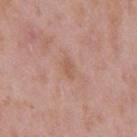The lesion was tiled from a total-body skin photograph and was not biopsied. A 15 mm close-up tile from a total-body photography series done for melanoma screening. The lesion's longest dimension is about 2.5 mm. From the back. A male subject about 55 years old. Captured under white-light illumination. The lesion-visualizer software estimated a footprint of about 3 mm², an outline eccentricity of about 0.85 (0 = round, 1 = elongated), and a symmetry-axis asymmetry near 0.2. The analysis additionally found a lesion color around L≈57 a*≈21 b*≈29 in CIELAB, about 6 CIELAB-L* units darker than the surrounding skin, and a normalized border contrast of about 5.5. The software also gave border irregularity of about 2 on a 0–10 scale, internal color variation of about 0.5 on a 0–10 scale, and peripheral color asymmetry of about 0.